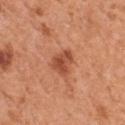This image is a 15 mm lesion crop taken from a total-body photograph.
The subject is a male aged around 55.
The lesion is located on the chest.
The tile uses white-light illumination.